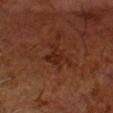Clinical impression:
Recorded during total-body skin imaging; not selected for excision or biopsy.
Acquisition and patient details:
A male subject, in their mid- to late 60s. A lesion tile, about 15 mm wide, cut from a 3D total-body photograph. Imaged with cross-polarized lighting.